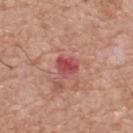Q: Was a biopsy performed?
A: total-body-photography surveillance lesion; no biopsy
Q: What lighting was used for the tile?
A: white-light
Q: Lesion size?
A: ≈3 mm
Q: What kind of image is this?
A: ~15 mm tile from a whole-body skin photo
Q: Lesion location?
A: the upper back
Q: Patient demographics?
A: male, about 60 years old